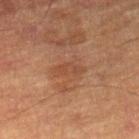No biopsy was performed on this lesion — it was imaged during a full skin examination and was not determined to be concerning. The patient is a male in their mid- to late 80s or older. A region of skin cropped from a whole-body photographic capture, roughly 15 mm wide. The lesion is located on the leg.